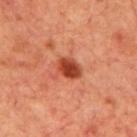Q: Is there a histopathology result?
A: no biopsy performed (imaged during a skin exam)
Q: What is the anatomic site?
A: the back
Q: What is the imaging modality?
A: 15 mm crop, total-body photography
Q: What lighting was used for the tile?
A: cross-polarized illumination
Q: What are the patient's age and sex?
A: male, aged 68–72